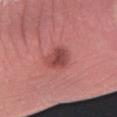  biopsy_status: not biopsied; imaged during a skin examination